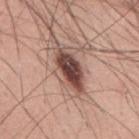Q: Was a biopsy performed?
A: catalogued during a skin exam; not biopsied
Q: Lesion location?
A: the mid back
Q: What kind of image is this?
A: 15 mm crop, total-body photography
Q: Who is the patient?
A: male, aged approximately 60
Q: How was the tile lit?
A: white-light
Q: What did automated image analysis measure?
A: a footprint of about 13 mm², a shape eccentricity near 0.85, and a shape-asymmetry score of about 0.25 (0 = symmetric); about 18 CIELAB-L* units darker than the surrounding skin and a normalized border contrast of about 12
Q: What is the lesion's diameter?
A: ~6 mm (longest diameter)